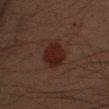Imaged during a routine full-body skin examination; the lesion was not biopsied and no histopathology is available. A male subject, in their mid- to late 40s. A roughly 15 mm field-of-view crop from a total-body skin photograph. On the arm.